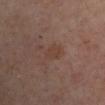{
  "biopsy_status": "not biopsied; imaged during a skin examination",
  "lighting": "cross-polarized",
  "lesion_size": {
    "long_diameter_mm_approx": 3.0
  },
  "site": "right upper arm",
  "patient": {
    "age_approx": 55
  },
  "image": {
    "source": "total-body photography crop",
    "field_of_view_mm": 15
  }
}